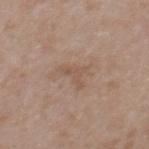Impression: The lesion was tiled from a total-body skin photograph and was not biopsied. Clinical summary: On the back. A female subject in their 30s. The lesion's longest dimension is about 3.5 mm. The lesion-visualizer software estimated a lesion color around L≈53 a*≈17 b*≈28 in CIELAB and a lesion–skin lightness drop of about 6. The software also gave a detector confidence of about 100 out of 100 that the crop contains a lesion. A close-up tile cropped from a whole-body skin photograph, about 15 mm across. The tile uses white-light illumination.